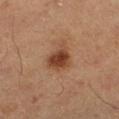Assessment: Imaged during a routine full-body skin examination; the lesion was not biopsied and no histopathology is available. Acquisition and patient details: The lesion's longest dimension is about 3.5 mm. A 15 mm crop from a total-body photograph taken for skin-cancer surveillance. Imaged with cross-polarized lighting. A male patient in their 60s. On the left lower leg.